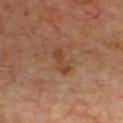Image and clinical context:
From the chest. A male subject approximately 70 years of age. Cropped from a total-body skin-imaging series; the visible field is about 15 mm. The tile uses cross-polarized illumination. The lesion's longest dimension is about 3 mm.